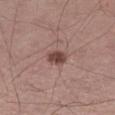Case summary:
– automated metrics: a mean CIELAB color near L≈44 a*≈20 b*≈23 and about 13 CIELAB-L* units darker than the surrounding skin; a nevus-likeness score of about 95/100 and a lesion-detection confidence of about 100/100
– patient: male, aged approximately 55
– tile lighting: white-light illumination
– site: the right thigh
– lesion diameter: ~2.5 mm (longest diameter)
– imaging modality: total-body-photography crop, ~15 mm field of view